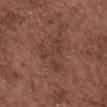Part of a total-body skin-imaging series; this lesion was reviewed on a skin check and was not flagged for biopsy. The total-body-photography lesion software estimated an area of roughly 9 mm², an eccentricity of roughly 0.7, and two-axis asymmetry of about 0.8. It also reported a lesion color around L≈38 a*≈19 b*≈24 in CIELAB, a lesion–skin lightness drop of about 6, and a normalized lesion–skin contrast near 5.5. It also reported a border-irregularity rating of about 10/10 and radial color variation of about 0.5. The software also gave a classifier nevus-likeness of about 0/100 and a detector confidence of about 90 out of 100 that the crop contains a lesion. This is a white-light tile. On the chest. The lesion's longest dimension is about 5 mm. A male subject, aged approximately 75. A 15 mm close-up extracted from a 3D total-body photography capture.